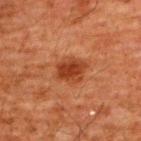Findings:
• image: 15 mm crop, total-body photography
• anatomic site: the back
• diameter: ~3.5 mm (longest diameter)
• illumination: cross-polarized illumination
• patient: male, aged 58 to 62